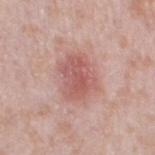workup: total-body-photography surveillance lesion; no biopsy | acquisition: total-body-photography crop, ~15 mm field of view | anatomic site: the left thigh | patient: female, about 60 years old.